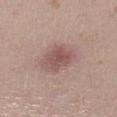| feature | finding |
|---|---|
| imaging modality | 15 mm crop, total-body photography |
| subject | female, aged approximately 20 |
| size | about 4.5 mm |
| tile lighting | white-light |
| anatomic site | the leg |